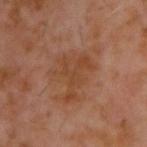Q: Is there a histopathology result?
A: catalogued during a skin exam; not biopsied